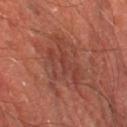Q: Who is the patient?
A: male, approximately 65 years of age
Q: What is the imaging modality?
A: ~15 mm tile from a whole-body skin photo
Q: What is the anatomic site?
A: the left lower leg